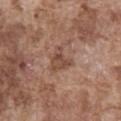Impression: Part of a total-body skin-imaging series; this lesion was reviewed on a skin check and was not flagged for biopsy. Clinical summary: Approximately 3.5 mm at its widest. The lesion is located on the abdomen. Cropped from a total-body skin-imaging series; the visible field is about 15 mm. Imaged with white-light lighting. A male subject in their mid- to late 70s. Automated image analysis of the tile measured a within-lesion color-variation index near 4/10 and a peripheral color-asymmetry measure near 1.5.